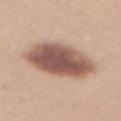This lesion was catalogued during total-body skin photography and was not selected for biopsy.
Longest diameter approximately 8 mm.
A 15 mm close-up tile from a total-body photography series done for melanoma screening.
A female patient, in their mid-30s.
Imaged with white-light lighting.
Located on the back.